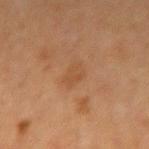| key | value |
|---|---|
| notes | total-body-photography surveillance lesion; no biopsy |
| automated lesion analysis | lesion-presence confidence of about 100/100 |
| image | total-body-photography crop, ~15 mm field of view |
| site | the left forearm |
| lighting | cross-polarized |
| lesion diameter | ≈2.5 mm |
| patient | female, aged approximately 40 |